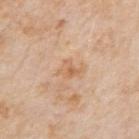A male patient aged 73 to 77. The tile uses white-light illumination. Cropped from a whole-body photographic skin survey; the tile spans about 15 mm. Approximately 2.5 mm at its widest. Located on the right upper arm.